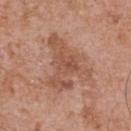follow-up: imaged on a skin check; not biopsied | illumination: white-light | diameter: about 6.5 mm | acquisition: 15 mm crop, total-body photography | anatomic site: the chest | subject: male, aged approximately 70.